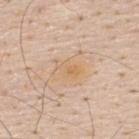Part of a total-body skin-imaging series; this lesion was reviewed on a skin check and was not flagged for biopsy.
The lesion is on the upper back.
A region of skin cropped from a whole-body photographic capture, roughly 15 mm wide.
Imaged with white-light lighting.
Approximately 3 mm at its widest.
A male patient, in their 60s.